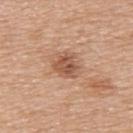Recorded during total-body skin imaging; not selected for excision or biopsy. Located on the upper back. The subject is a male aged 68 to 72. This is a white-light tile. The recorded lesion diameter is about 3.5 mm. This image is a 15 mm lesion crop taken from a total-body photograph. The lesion-visualizer software estimated a footprint of about 7 mm², an eccentricity of roughly 0.45, and a symmetry-axis asymmetry near 0.25. It also reported an average lesion color of about L≈54 a*≈22 b*≈32 (CIELAB), roughly 11 lightness units darker than nearby skin, and a lesion-to-skin contrast of about 7.5 (normalized; higher = more distinct). And it measured a lesion-detection confidence of about 100/100.